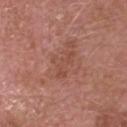workup: catalogued during a skin exam; not biopsied | TBP lesion metrics: a mean CIELAB color near L≈49 a*≈24 b*≈28, roughly 6 lightness units darker than nearby skin, and a lesion-to-skin contrast of about 5 (normalized; higher = more distinct) | acquisition: ~15 mm crop, total-body skin-cancer survey | subject: male, aged 73 to 77 | lesion size: ≈4 mm | lighting: white-light | anatomic site: the head or neck.